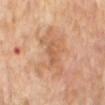Recorded during total-body skin imaging; not selected for excision or biopsy.
The tile uses cross-polarized illumination.
A male patient, aged 63–67.
From the back.
Automated image analysis of the tile measured border irregularity of about 7.5 on a 0–10 scale and a within-lesion color-variation index near 3/10. It also reported a nevus-likeness score of about 0/100.
Approximately 6.5 mm at its widest.
Cropped from a total-body skin-imaging series; the visible field is about 15 mm.